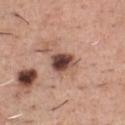biopsy status: no biopsy performed (imaged during a skin exam) | imaging modality: ~15 mm tile from a whole-body skin photo | illumination: white-light | diameter: ~3 mm (longest diameter) | site: the chest | TBP lesion metrics: a footprint of about 6.5 mm² and a shape eccentricity near 0.65; a mean CIELAB color near L≈45 a*≈21 b*≈24, roughly 19 lightness units darker than nearby skin, and a lesion-to-skin contrast of about 13 (normalized; higher = more distinct); a border-irregularity index near 2/10, internal color variation of about 7 on a 0–10 scale, and radial color variation of about 2; lesion-presence confidence of about 100/100 | subject: male, aged around 45.